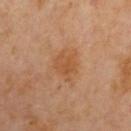Case summary:
• follow-up · catalogued during a skin exam; not biopsied
• diameter · ~3.5 mm (longest diameter)
• tile lighting · cross-polarized
• TBP lesion metrics · a footprint of about 6.5 mm², a shape eccentricity near 0.6, and a shape-asymmetry score of about 0.25 (0 = symmetric); a border-irregularity rating of about 3/10, a within-lesion color-variation index near 2.5/10, and radial color variation of about 1
• image · ~15 mm tile from a whole-body skin photo
• subject · male, about 60 years old
• anatomic site · the left upper arm